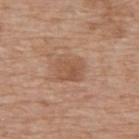The lesion was photographed on a routine skin check and not biopsied; there is no pathology result.
On the upper back.
A 15 mm crop from a total-body photograph taken for skin-cancer surveillance.
A female patient approximately 75 years of age.